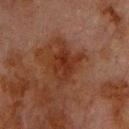Clinical impression: The lesion was photographed on a routine skin check and not biopsied; there is no pathology result. Image and clinical context: About 4.5 mm across. Cropped from a whole-body photographic skin survey; the tile spans about 15 mm. A male patient, aged around 80. From the chest. Imaged with cross-polarized lighting.